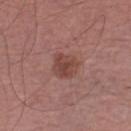Assessment:
Recorded during total-body skin imaging; not selected for excision or biopsy.
Background:
This image is a 15 mm lesion crop taken from a total-body photograph. From the left lower leg. The tile uses white-light illumination. The subject is a male approximately 65 years of age. Approximately 3 mm at its widest.